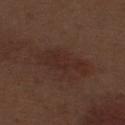Impression: The lesion was tiled from a total-body skin photograph and was not biopsied. Background: Captured under white-light illumination. The recorded lesion diameter is about 6 mm. This image is a 15 mm lesion crop taken from a total-body photograph. The lesion-visualizer software estimated a footprint of about 10 mm² and two-axis asymmetry of about 0.35. The analysis additionally found an average lesion color of about L≈27 a*≈18 b*≈22 (CIELAB), roughly 5 lightness units darker than nearby skin, and a lesion-to-skin contrast of about 6 (normalized; higher = more distinct). The analysis additionally found border irregularity of about 5.5 on a 0–10 scale, internal color variation of about 2 on a 0–10 scale, and radial color variation of about 0.5. The software also gave a nevus-likeness score of about 5/100 and a lesion-detection confidence of about 100/100. A male subject aged approximately 70. On the left thigh.